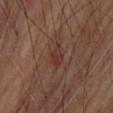No biopsy was performed on this lesion — it was imaged during a full skin examination and was not determined to be concerning. The total-body-photography lesion software estimated a mean CIELAB color near L≈34 a*≈22 b*≈24, a lesion–skin lightness drop of about 5, and a normalized lesion–skin contrast near 5. And it measured a border-irregularity index near 3.5/10, internal color variation of about 2.5 on a 0–10 scale, and radial color variation of about 1. Cropped from a total-body skin-imaging series; the visible field is about 15 mm. A male patient in their mid-60s. On the upper back. Longest diameter approximately 3 mm.